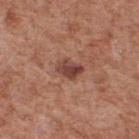<lesion>
<biopsy_status>not biopsied; imaged during a skin examination</biopsy_status>
<image>
  <source>total-body photography crop</source>
  <field_of_view_mm>15</field_of_view_mm>
</image>
<patient>
  <sex>male</sex>
  <age_approx>65</age_approx>
</patient>
<site>upper back</site>
<lesion_size>
  <long_diameter_mm_approx>3.0</long_diameter_mm_approx>
</lesion_size>
<lighting>white-light</lighting>
</lesion>